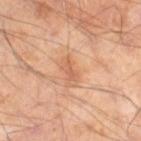- follow-up: catalogued during a skin exam; not biopsied
- lesion size: ~3 mm (longest diameter)
- lighting: cross-polarized
- body site: the right thigh
- subject: male, aged around 65
- acquisition: 15 mm crop, total-body photography